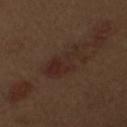Q: Was this lesion biopsied?
A: no biopsy performed (imaged during a skin exam)
Q: What is the imaging modality?
A: 15 mm crop, total-body photography
Q: What is the anatomic site?
A: the right upper arm
Q: What are the patient's age and sex?
A: male, aged 68 to 72